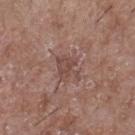Impression:
This lesion was catalogued during total-body skin photography and was not selected for biopsy.
Acquisition and patient details:
Longest diameter approximately 3 mm. A female patient, in their mid- to late 70s. Imaged with white-light lighting. The total-body-photography lesion software estimated a lesion area of about 6 mm², an eccentricity of roughly 0.65, and a shape-asymmetry score of about 0.4 (0 = symmetric). The analysis additionally found a mean CIELAB color near L≈46 a*≈19 b*≈22, roughly 7 lightness units darker than nearby skin, and a lesion-to-skin contrast of about 6 (normalized; higher = more distinct). This image is a 15 mm lesion crop taken from a total-body photograph. The lesion is on the right lower leg.